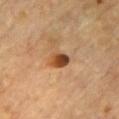The lesion was photographed on a routine skin check and not biopsied; there is no pathology result.
A region of skin cropped from a whole-body photographic capture, roughly 15 mm wide.
A male patient, aged approximately 85 or older.
Approximately 2.5 mm at its widest.
The lesion is on the front of the torso.